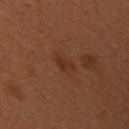{
  "biopsy_status": "not biopsied; imaged during a skin examination",
  "patient": {
    "sex": "female",
    "age_approx": 50
  },
  "lesion_size": {
    "long_diameter_mm_approx": 2.5
  },
  "lighting": "cross-polarized",
  "image": {
    "source": "total-body photography crop",
    "field_of_view_mm": 15
  },
  "site": "arm"
}